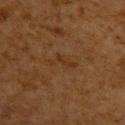No biopsy was performed on this lesion — it was imaged during a full skin examination and was not determined to be concerning.
Approximately 4 mm at its widest.
The lesion is on the upper back.
A male patient about 60 years old.
A 15 mm crop from a total-body photograph taken for skin-cancer surveillance.
This is a cross-polarized tile.